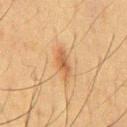notes=catalogued during a skin exam; not biopsied | size=~3.5 mm (longest diameter) | patient=male, in their 60s | lighting=cross-polarized illumination | anatomic site=the chest | image=~15 mm tile from a whole-body skin photo.